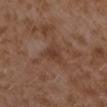Clinical impression:
Imaged during a routine full-body skin examination; the lesion was not biopsied and no histopathology is available.
Image and clinical context:
A female subject in their mid-30s. The lesion is on the left forearm. A lesion tile, about 15 mm wide, cut from a 3D total-body photograph.